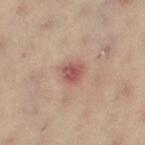Recorded during total-body skin imaging; not selected for excision or biopsy.
This image is a 15 mm lesion crop taken from a total-body photograph.
The lesion is located on the right thigh.
The subject is a female in their mid- to late 40s.
The total-body-photography lesion software estimated a mean CIELAB color near L≈53 a*≈24 b*≈24. It also reported a within-lesion color-variation index near 3.5/10. It also reported an automated nevus-likeness rating near 5 out of 100 and a detector confidence of about 100 out of 100 that the crop contains a lesion.
The lesion's longest dimension is about 2.5 mm.